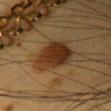notes: imaged on a skin check; not biopsied | lesion size: ≈5.5 mm | patient: female, aged 28–32 | acquisition: ~15 mm crop, total-body skin-cancer survey | site: the head or neck.